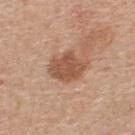<case>
<biopsy_status>not biopsied; imaged during a skin examination</biopsy_status>
<lighting>white-light</lighting>
<image>
  <source>total-body photography crop</source>
  <field_of_view_mm>15</field_of_view_mm>
</image>
<site>upper back</site>
<patient>
  <sex>male</sex>
  <age_approx>55</age_approx>
</patient>
<lesion_size>
  <long_diameter_mm_approx>5.0</long_diameter_mm_approx>
</lesion_size>
<automated_metrics>
  <area_mm2_approx>11.0</area_mm2_approx>
  <eccentricity>0.7</eccentricity>
  <cielab_L>53</cielab_L>
  <cielab_a>22</cielab_a>
  <cielab_b>32</cielab_b>
  <vs_skin_darker_L>12.0</vs_skin_darker_L>
  <vs_skin_contrast_norm>8.0</vs_skin_contrast_norm>
  <border_irregularity_0_10>2.5</border_irregularity_0_10>
  <color_variation_0_10>2.5</color_variation_0_10>
  <peripheral_color_asymmetry>1.0</peripheral_color_asymmetry>
</automated_metrics>
</case>